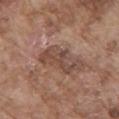Captured during whole-body skin photography for melanoma surveillance; the lesion was not biopsied. The lesion-visualizer software estimated a border-irregularity rating of about 5.5/10, a within-lesion color-variation index near 4/10, and a peripheral color-asymmetry measure near 1. It also reported a nevus-likeness score of about 0/100 and lesion-presence confidence of about 95/100. A male patient, roughly 75 years of age. About 6 mm across. A 15 mm close-up extracted from a 3D total-body photography capture. The tile uses white-light illumination. From the mid back.